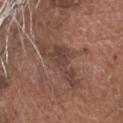This lesion was catalogued during total-body skin photography and was not selected for biopsy.
A male subject, about 75 years old.
On the head or neck.
About 6.5 mm across.
A region of skin cropped from a whole-body photographic capture, roughly 15 mm wide.
The total-body-photography lesion software estimated about 8 CIELAB-L* units darker than the surrounding skin and a normalized border contrast of about 6.5. And it measured a nevus-likeness score of about 0/100 and a detector confidence of about 90 out of 100 that the crop contains a lesion.